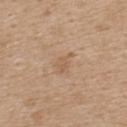Recorded during total-body skin imaging; not selected for excision or biopsy. Cropped from a whole-body photographic skin survey; the tile spans about 15 mm. About 3 mm across. The total-body-photography lesion software estimated a lesion area of about 3.5 mm², an eccentricity of roughly 0.8, and two-axis asymmetry of about 0.4. It also reported a lesion color around L≈58 a*≈17 b*≈33 in CIELAB. The analysis additionally found a peripheral color-asymmetry measure near 0.5. And it measured a nevus-likeness score of about 0/100 and a lesion-detection confidence of about 100/100. The subject is a female roughly 45 years of age. Located on the upper back.